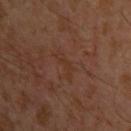Clinical summary:
The lesion is on the arm. A male subject, aged 28–32. A region of skin cropped from a whole-body photographic capture, roughly 15 mm wide.